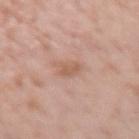On the left forearm. The lesion's longest dimension is about 2.5 mm. A female patient, approximately 40 years of age. A lesion tile, about 15 mm wide, cut from a 3D total-body photograph.